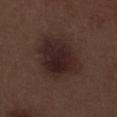workup = imaged on a skin check; not biopsied | imaging modality = ~15 mm tile from a whole-body skin photo | lighting = white-light | site = the right lower leg | size = ≈6.5 mm | patient = male, approximately 70 years of age.